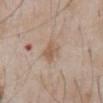notes — no biopsy performed (imaged during a skin exam)
patient — male, aged approximately 60
lighting — white-light
automated lesion analysis — a lesion color around L≈58 a*≈15 b*≈29 in CIELAB, a lesion–skin lightness drop of about 8, and a lesion-to-skin contrast of about 6 (normalized; higher = more distinct); a border-irregularity index near 2.5/10, a within-lesion color-variation index near 3/10, and a peripheral color-asymmetry measure near 1; a detector confidence of about 100 out of 100 that the crop contains a lesion
lesion diameter — ≈3 mm
location — the abdomen
image — ~15 mm crop, total-body skin-cancer survey